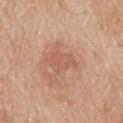Assessment: The lesion was tiled from a total-body skin photograph and was not biopsied. Context: On the back. A male subject, about 80 years old. A 15 mm close-up tile from a total-body photography series done for melanoma screening. Approximately 4.5 mm at its widest.